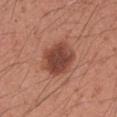The lesion was tiled from a total-body skin photograph and was not biopsied.
Cropped from a total-body skin-imaging series; the visible field is about 15 mm.
Automated tile analysis of the lesion measured a normalized lesion–skin contrast near 9.5. The analysis additionally found an automated nevus-likeness rating near 95 out of 100 and lesion-presence confidence of about 100/100.
The patient is a male approximately 35 years of age.
Imaged with white-light lighting.
On the left upper arm.